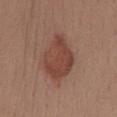biopsy_status: not biopsied; imaged during a skin examination
patient:
  sex: female
  age_approx: 55
lighting: white-light
site: mid back
image:
  source: total-body photography crop
  field_of_view_mm: 15
automated_metrics:
  vs_skin_darker_L: 9.0
  vs_skin_contrast_norm: 7.5
  border_irregularity_0_10: 2.0
  nevus_likeness_0_100: 85
  lesion_detection_confidence_0_100: 100